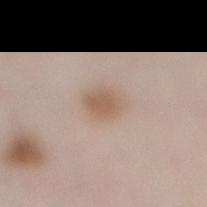Assessment: The lesion was tiled from a total-body skin photograph and was not biopsied. Context: The recorded lesion diameter is about 3 mm. Captured under white-light illumination. This image is a 15 mm lesion crop taken from a total-body photograph. The lesion is on the leg. A female patient, in their 60s.